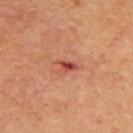Case summary:
– workup: no biopsy performed (imaged during a skin exam)
– diameter: about 2.5 mm
– acquisition: 15 mm crop, total-body photography
– anatomic site: the upper back
– patient: male, aged approximately 65